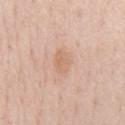notes=catalogued during a skin exam; not biopsied
diameter=≈3.5 mm
location=the chest
subject=male, aged 58 to 62
image=~15 mm tile from a whole-body skin photo
tile lighting=white-light
automated lesion analysis=an area of roughly 6 mm² and a shape eccentricity near 0.75; border irregularity of about 2 on a 0–10 scale and radial color variation of about 1; a nevus-likeness score of about 0/100 and a lesion-detection confidence of about 100/100